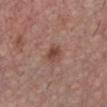A region of skin cropped from a whole-body photographic capture, roughly 15 mm wide. An algorithmic analysis of the crop reported a footprint of about 4.5 mm², an eccentricity of roughly 0.75, and two-axis asymmetry of about 0.25. The software also gave an average lesion color of about L≈46 a*≈21 b*≈26 (CIELAB) and a lesion-to-skin contrast of about 7.5 (normalized; higher = more distinct). And it measured lesion-presence confidence of about 100/100. Imaged with white-light lighting. The lesion is located on the chest. The subject is a male aged around 75. Longest diameter approximately 3 mm.